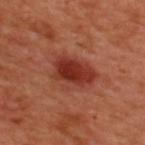The lesion was tiled from a total-body skin photograph and was not biopsied.
Captured under cross-polarized illumination.
Located on the upper back.
Cropped from a total-body skin-imaging series; the visible field is about 15 mm.
Automated image analysis of the tile measured an area of roughly 14 mm², a shape eccentricity near 0.75, and a symmetry-axis asymmetry near 0.2. It also reported a mean CIELAB color near L≈37 a*≈31 b*≈30, about 11 CIELAB-L* units darker than the surrounding skin, and a normalized border contrast of about 9.
About 5.5 mm across.
A male subject, approximately 50 years of age.